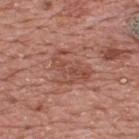notes = catalogued during a skin exam; not biopsied | image = ~15 mm tile from a whole-body skin photo | patient = male, roughly 70 years of age | location = the upper back | lighting = white-light illumination | size = about 5 mm.